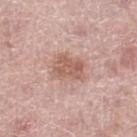- follow-up — no biopsy performed (imaged during a skin exam)
- lesion size — ≈3.5 mm
- imaging modality — ~15 mm crop, total-body skin-cancer survey
- subject — female, aged 58–62
- body site — the leg
- automated metrics — a shape eccentricity near 0.65 and two-axis asymmetry of about 0.3; a lesion color around L≈59 a*≈21 b*≈26 in CIELAB, a lesion–skin lightness drop of about 10, and a lesion-to-skin contrast of about 7 (normalized; higher = more distinct); a border-irregularity rating of about 3/10 and a color-variation rating of about 2.5/10; an automated nevus-likeness rating near 30 out of 100 and a lesion-detection confidence of about 100/100